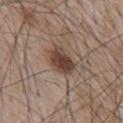Impression: This lesion was catalogued during total-body skin photography and was not selected for biopsy. Image and clinical context: A male patient, roughly 65 years of age. About 4 mm across. This is a white-light tile. Automated tile analysis of the lesion measured a lesion color around L≈42 a*≈17 b*≈25 in CIELAB and about 13 CIELAB-L* units darker than the surrounding skin. The analysis additionally found internal color variation of about 4 on a 0–10 scale and radial color variation of about 1.5. A region of skin cropped from a whole-body photographic capture, roughly 15 mm wide. From the chest.